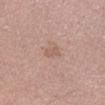Image and clinical context: A male subject, aged approximately 40. Captured under white-light illumination. A region of skin cropped from a whole-body photographic capture, roughly 15 mm wide. An algorithmic analysis of the crop reported a lesion color around L≈58 a*≈19 b*≈26 in CIELAB, roughly 7 lightness units darker than nearby skin, and a normalized lesion–skin contrast near 4.5. The software also gave border irregularity of about 2.5 on a 0–10 scale, a within-lesion color-variation index near 1/10, and peripheral color asymmetry of about 0.5. The analysis additionally found a nevus-likeness score of about 0/100 and a detector confidence of about 100 out of 100 that the crop contains a lesion. From the right lower leg. The lesion's longest dimension is about 2.5 mm.